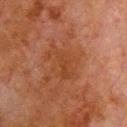This lesion was catalogued during total-body skin photography and was not selected for biopsy.
The lesion is on the chest.
The lesion's longest dimension is about 4.5 mm.
A region of skin cropped from a whole-body photographic capture, roughly 15 mm wide.
The patient is a male aged approximately 80.